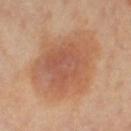{"biopsy_status": "not biopsied; imaged during a skin examination", "lighting": "cross-polarized", "patient": {"sex": "female", "age_approx": 45}, "site": "left thigh", "image": {"source": "total-body photography crop", "field_of_view_mm": 15}}